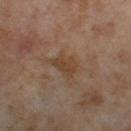Assessment:
Imaged during a routine full-body skin examination; the lesion was not biopsied and no histopathology is available.
Image and clinical context:
A lesion tile, about 15 mm wide, cut from a 3D total-body photograph. The lesion's longest dimension is about 3 mm. Imaged with cross-polarized lighting. On the right thigh. The patient is a female aged 53–57.